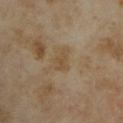Impression:
Imaged during a routine full-body skin examination; the lesion was not biopsied and no histopathology is available.